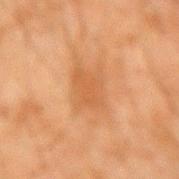The lesion was tiled from a total-body skin photograph and was not biopsied.
The total-body-photography lesion software estimated an average lesion color of about L≈49 a*≈20 b*≈35 (CIELAB), about 6 CIELAB-L* units darker than the surrounding skin, and a normalized border contrast of about 5. It also reported an automated nevus-likeness rating near 0 out of 100 and a lesion-detection confidence of about 100/100.
Longest diameter approximately 6 mm.
Captured under cross-polarized illumination.
A close-up tile cropped from a whole-body skin photograph, about 15 mm across.
A male subject, aged approximately 45.
On the right forearm.